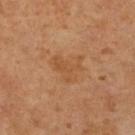workup: total-body-photography surveillance lesion; no biopsy | lighting: cross-polarized illumination | imaging modality: ~15 mm crop, total-body skin-cancer survey | site: the leg | subject: female, aged 63–67 | lesion diameter: ~3.5 mm (longest diameter).